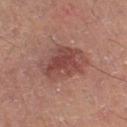Imaged during a routine full-body skin examination; the lesion was not biopsied and no histopathology is available. The lesion-visualizer software estimated a lesion area of about 16 mm², an outline eccentricity of about 0.5 (0 = round, 1 = elongated), and a symmetry-axis asymmetry near 0.3. The software also gave a border-irregularity rating of about 3.5/10, a color-variation rating of about 4/10, and a peripheral color-asymmetry measure near 1.5. Located on the leg. This is a cross-polarized tile. This image is a 15 mm lesion crop taken from a total-body photograph. The patient is a male in their 50s.